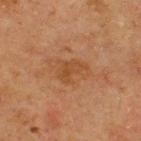workup = imaged on a skin check; not biopsied
subject = male, aged 68 to 72
image = ~15 mm crop, total-body skin-cancer survey
anatomic site = the upper back